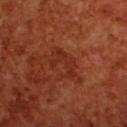Imaged during a routine full-body skin examination; the lesion was not biopsied and no histopathology is available.